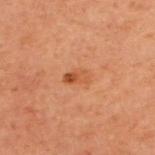follow-up: imaged on a skin check; not biopsied
patient: male, in their 60s
TBP lesion metrics: a lesion area of about 4.5 mm², an eccentricity of roughly 0.8, and a shape-asymmetry score of about 0.2 (0 = symmetric); roughly 7 lightness units darker than nearby skin and a normalized lesion–skin contrast near 6
lighting: cross-polarized
imaging modality: ~15 mm crop, total-body skin-cancer survey
lesion diameter: ~3 mm (longest diameter)
site: the upper back